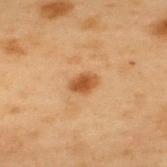Assessment:
The lesion was photographed on a routine skin check and not biopsied; there is no pathology result.
Context:
The lesion is located on the upper back. The tile uses cross-polarized illumination. A 15 mm close-up tile from a total-body photography series done for melanoma screening. A male patient roughly 55 years of age. Longest diameter approximately 2.5 mm.